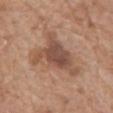Findings:
• notes · catalogued during a skin exam; not biopsied
• lighting · white-light
• TBP lesion metrics · a footprint of about 16 mm², an eccentricity of roughly 0.75, and a symmetry-axis asymmetry near 0.55
• lesion diameter · ≈6.5 mm
• site · the back
• imaging modality · ~15 mm tile from a whole-body skin photo
• subject · female, aged 73 to 77